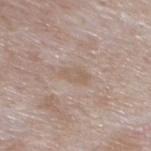Context:
Cropped from a whole-body photographic skin survey; the tile spans about 15 mm. A male subject, in their mid- to late 70s. The lesion is located on the mid back. This is a white-light tile. Longest diameter approximately 3 mm.